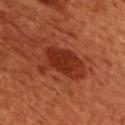Captured under cross-polarized illumination.
The recorded lesion diameter is about 6 mm.
A male patient, aged approximately 50.
Automated tile analysis of the lesion measured a lesion area of about 16 mm², an outline eccentricity of about 0.8 (0 = round, 1 = elongated), and a symmetry-axis asymmetry near 0.4.
A region of skin cropped from a whole-body photographic capture, roughly 15 mm wide.
On the chest.